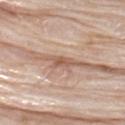* follow-up — imaged on a skin check; not biopsied
* lesion size — about 2.5 mm
* anatomic site — the upper back
* automated metrics — a lesion area of about 3 mm², a shape eccentricity near 0.9, and two-axis asymmetry of about 0.3
* acquisition — ~15 mm tile from a whole-body skin photo
* subject — male, approximately 75 years of age
* lighting — white-light illumination